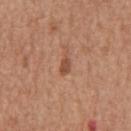biopsy status=catalogued during a skin exam; not biopsied | size=~2.5 mm (longest diameter) | image source=15 mm crop, total-body photography | site=the chest | lighting=white-light illumination | patient=male, roughly 65 years of age.